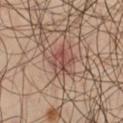{
  "biopsy_status": "not biopsied; imaged during a skin examination",
  "automated_metrics": {
    "eccentricity": 0.55,
    "shape_asymmetry": 0.25,
    "cielab_L": 46,
    "cielab_a": 21,
    "cielab_b": 24,
    "vs_skin_darker_L": 9.0,
    "vs_skin_contrast_norm": 7.0,
    "nevus_likeness_0_100": 0
  },
  "site": "left thigh",
  "lesion_size": {
    "long_diameter_mm_approx": 3.0
  },
  "image": {
    "source": "total-body photography crop",
    "field_of_view_mm": 15
  },
  "patient": {
    "sex": "male",
    "age_approx": 60
  }
}